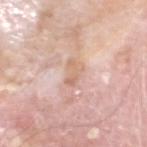Assessment: Recorded during total-body skin imaging; not selected for excision or biopsy. Background: The total-body-photography lesion software estimated a border-irregularity index near 4/10 and radial color variation of about 0.5. The software also gave lesion-presence confidence of about 100/100. This is a white-light tile. A region of skin cropped from a whole-body photographic capture, roughly 15 mm wide. About 3 mm across. A male patient, aged 73 to 77. On the head or neck.